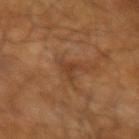No biopsy was performed on this lesion — it was imaged during a full skin examination and was not determined to be concerning. A close-up tile cropped from a whole-body skin photograph, about 15 mm across. A male patient aged around 65. Imaged with cross-polarized lighting.